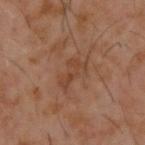This lesion was catalogued during total-body skin photography and was not selected for biopsy.
A male subject, aged 58–62.
A lesion tile, about 15 mm wide, cut from a 3D total-body photograph.
From the upper back.
The tile uses cross-polarized illumination.